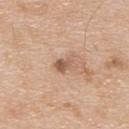{
  "biopsy_status": "not biopsied; imaged during a skin examination",
  "image": {
    "source": "total-body photography crop",
    "field_of_view_mm": 15
  },
  "patient": {
    "sex": "male",
    "age_approx": 65
  },
  "site": "upper back"
}